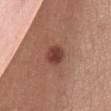<case>
  <image>
    <source>total-body photography crop</source>
    <field_of_view_mm>15</field_of_view_mm>
  </image>
  <lighting>white-light</lighting>
  <site>chest</site>
  <lesion_size>
    <long_diameter_mm_approx>3.0</long_diameter_mm_approx>
  </lesion_size>
  <patient>
    <sex>female</sex>
    <age_approx>40</age_approx>
  </patient>
</case>